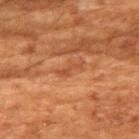biopsy status: imaged on a skin check; not biopsied | image: ~15 mm tile from a whole-body skin photo | site: the front of the torso | patient: female, about 80 years old.